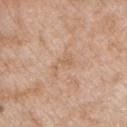Q: Patient demographics?
A: male, in their mid- to late 60s
Q: Illumination type?
A: white-light
Q: Where on the body is the lesion?
A: the chest
Q: What did automated image analysis measure?
A: a lesion color around L≈62 a*≈18 b*≈32 in CIELAB, about 6 CIELAB-L* units darker than the surrounding skin, and a lesion-to-skin contrast of about 4.5 (normalized; higher = more distinct); a nevus-likeness score of about 0/100 and a lesion-detection confidence of about 100/100
Q: How large is the lesion?
A: ≈3.5 mm
Q: What kind of image is this?
A: ~15 mm crop, total-body skin-cancer survey